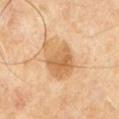Recorded during total-body skin imaging; not selected for excision or biopsy. The total-body-photography lesion software estimated a lesion color around L≈62 a*≈19 b*≈39 in CIELAB, about 10 CIELAB-L* units darker than the surrounding skin, and a lesion-to-skin contrast of about 7 (normalized; higher = more distinct). A male subject, aged approximately 60. The tile uses cross-polarized illumination. Located on the back. Approximately 5.5 mm at its widest. A roughly 15 mm field-of-view crop from a total-body skin photograph.